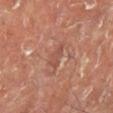<case>
  <biopsy_status>not biopsied; imaged during a skin examination</biopsy_status>
  <patient>
    <sex>male</sex>
    <age_approx>50</age_approx>
  </patient>
  <lighting>cross-polarized</lighting>
  <site>leg</site>
  <automated_metrics>
    <area_mm2_approx>4.0</area_mm2_approx>
    <eccentricity>0.8</eccentricity>
    <shape_asymmetry>0.35</shape_asymmetry>
  </automated_metrics>
  <image>
    <source>total-body photography crop</source>
    <field_of_view_mm>15</field_of_view_mm>
  </image>
</case>